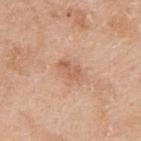The lesion was tiled from a total-body skin photograph and was not biopsied. Measured at roughly 3 mm in maximum diameter. Imaged with white-light lighting. A male subject, aged approximately 60. On the right upper arm. Automated tile analysis of the lesion measured a lesion color around L≈60 a*≈23 b*≈34 in CIELAB, roughly 8 lightness units darker than nearby skin, and a lesion-to-skin contrast of about 5.5 (normalized; higher = more distinct). The analysis additionally found a border-irregularity index near 3.5/10 and internal color variation of about 1.5 on a 0–10 scale. And it measured a lesion-detection confidence of about 100/100. A region of skin cropped from a whole-body photographic capture, roughly 15 mm wide.